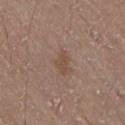Clinical impression:
Imaged during a routine full-body skin examination; the lesion was not biopsied and no histopathology is available.
Acquisition and patient details:
A 15 mm crop from a total-body photograph taken for skin-cancer surveillance. Located on the lower back. The recorded lesion diameter is about 3 mm. The subject is a male roughly 20 years of age.